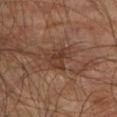<case>
<biopsy_status>not biopsied; imaged during a skin examination</biopsy_status>
<patient>
  <sex>male</sex>
  <age_approx>75</age_approx>
</patient>
<site>right upper arm</site>
<lesion_size>
  <long_diameter_mm_approx>3.5</long_diameter_mm_approx>
</lesion_size>
<lighting>cross-polarized</lighting>
<image>
  <source>total-body photography crop</source>
  <field_of_view_mm>15</field_of_view_mm>
</image>
</case>